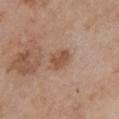notes — no biopsy performed (imaged during a skin exam)
site — the front of the torso
image-analysis metrics — a lesion-to-skin contrast of about 7 (normalized; higher = more distinct)
patient — female, in their mid- to late 60s
illumination — white-light illumination
lesion diameter — ~3.5 mm (longest diameter)
image — ~15 mm tile from a whole-body skin photo